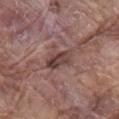No biopsy was performed on this lesion — it was imaged during a full skin examination and was not determined to be concerning. The lesion's longest dimension is about 3.5 mm. A 15 mm close-up tile from a total-body photography series done for melanoma screening. The tile uses white-light illumination. The lesion is on the mid back. The total-body-photography lesion software estimated a lesion–skin lightness drop of about 11 and a lesion-to-skin contrast of about 8.5 (normalized; higher = more distinct). It also reported a classifier nevus-likeness of about 5/100. A male subject approximately 80 years of age.